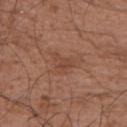notes=imaged on a skin check; not biopsied
illumination=white-light illumination
TBP lesion metrics=a lesion area of about 4.5 mm² and a shape eccentricity near 0.65; a mean CIELAB color near L≈45 a*≈21 b*≈29 and a normalized border contrast of about 5; a border-irregularity rating of about 5/10 and radial color variation of about 1
subject=male, aged 48–52
site=the left upper arm
size=about 3 mm
image=~15 mm tile from a whole-body skin photo